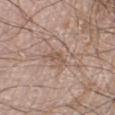No biopsy was performed on this lesion — it was imaged during a full skin examination and was not determined to be concerning.
Cropped from a whole-body photographic skin survey; the tile spans about 15 mm.
The tile uses white-light illumination.
On the leg.
A male patient aged 58–62.
Measured at roughly 3 mm in maximum diameter.